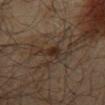This lesion was catalogued during total-body skin photography and was not selected for biopsy. On the mid back. The subject is a male aged 63 to 67. Captured under cross-polarized illumination. Automated image analysis of the tile measured a mean CIELAB color near L≈26 a*≈14 b*≈22, a lesion–skin lightness drop of about 6, and a lesion-to-skin contrast of about 7.5 (normalized; higher = more distinct). It also reported border irregularity of about 3.5 on a 0–10 scale, a color-variation rating of about 2.5/10, and a peripheral color-asymmetry measure near 1. The analysis additionally found a classifier nevus-likeness of about 0/100. About 2.5 mm across. A region of skin cropped from a whole-body photographic capture, roughly 15 mm wide.